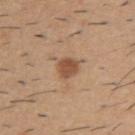Clinical impression: Recorded during total-body skin imaging; not selected for excision or biopsy. Background: The subject is a male in their 60s. Located on the back. A roughly 15 mm field-of-view crop from a total-body skin photograph.